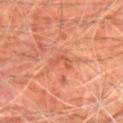No biopsy was performed on this lesion — it was imaged during a full skin examination and was not determined to be concerning.